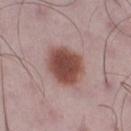follow-up: no biopsy performed (imaged during a skin exam) | anatomic site: the right thigh | patient: male, aged 48 to 52 | automated metrics: a lesion area of about 15 mm², a shape eccentricity near 0.4, and a shape-asymmetry score of about 0.15 (0 = symmetric); a border-irregularity index near 1.5/10, a color-variation rating of about 3.5/10, and a peripheral color-asymmetry measure near 1 | illumination: white-light illumination | size: ≈4.5 mm | image source: 15 mm crop, total-body photography.